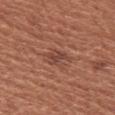biopsy status: catalogued during a skin exam; not biopsied
image: total-body-photography crop, ~15 mm field of view
body site: the right upper arm
lesion diameter: about 3 mm
image-analysis metrics: a lesion area of about 5 mm² and an eccentricity of roughly 0.6; an average lesion color of about L≈44 a*≈24 b*≈27 (CIELAB) and roughly 7 lightness units darker than nearby skin; a border-irregularity index near 3.5/10; lesion-presence confidence of about 100/100
lighting: white-light illumination
subject: female, aged 53 to 57